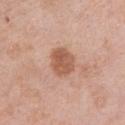Assessment: Recorded during total-body skin imaging; not selected for excision or biopsy. Acquisition and patient details: A female subject in their 50s. A 15 mm crop from a total-body photograph taken for skin-cancer surveillance. Approximately 3.5 mm at its widest. From the chest.